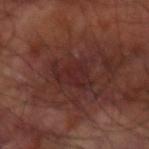The lesion is located on the right arm.
Automated image analysis of the tile measured about 5 CIELAB-L* units darker than the surrounding skin and a normalized border contrast of about 5.5. It also reported a border-irregularity index near 5.5/10, internal color variation of about 2.5 on a 0–10 scale, and peripheral color asymmetry of about 1. And it measured a classifier nevus-likeness of about 0/100 and a lesion-detection confidence of about 95/100.
A male patient, approximately 60 years of age.
The lesion's longest dimension is about 4.5 mm.
This image is a 15 mm lesion crop taken from a total-body photograph.